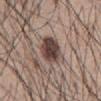Q: Lesion location?
A: the mid back
Q: What is the imaging modality?
A: ~15 mm tile from a whole-body skin photo
Q: How was the tile lit?
A: white-light
Q: Who is the patient?
A: male, aged 43 to 47